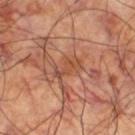Imaged during a routine full-body skin examination; the lesion was not biopsied and no histopathology is available.
A region of skin cropped from a whole-body photographic capture, roughly 15 mm wide.
Measured at roughly 3.5 mm in maximum diameter.
A male subject, roughly 60 years of age.
From the leg.